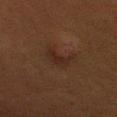follow-up: catalogued during a skin exam; not biopsied | patient: female, approximately 40 years of age | lighting: cross-polarized | imaging modality: ~15 mm crop, total-body skin-cancer survey | TBP lesion metrics: an average lesion color of about L≈20 a*≈15 b*≈21 (CIELAB), roughly 5 lightness units darker than nearby skin, and a normalized lesion–skin contrast near 6; an automated nevus-likeness rating near 5 out of 100 | diameter: ~3.5 mm (longest diameter) | location: the head or neck.